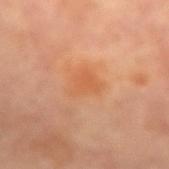Q: What is the anatomic site?
A: the left forearm
Q: What is the imaging modality?
A: ~15 mm tile from a whole-body skin photo
Q: Illumination type?
A: cross-polarized illumination
Q: What did automated image analysis measure?
A: an average lesion color of about L≈58 a*≈26 b*≈40 (CIELAB), a lesion–skin lightness drop of about 6, and a normalized lesion–skin contrast near 5.5; an automated nevus-likeness rating near 5 out of 100 and lesion-presence confidence of about 100/100
Q: Lesion size?
A: ≈3.5 mm
Q: Who is the patient?
A: female, about 65 years old